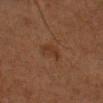Part of a total-body skin-imaging series; this lesion was reviewed on a skin check and was not flagged for biopsy.
Imaged with cross-polarized lighting.
A female subject aged around 40.
On the head or neck.
A 15 mm crop from a total-body photograph taken for skin-cancer surveillance.